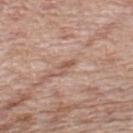Impression: Imaged during a routine full-body skin examination; the lesion was not biopsied and no histopathology is available.